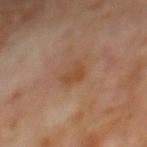Imaged during a routine full-body skin examination; the lesion was not biopsied and no histopathology is available.
From the mid back.
A male subject aged approximately 70.
This image is a 15 mm lesion crop taken from a total-body photograph.